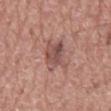No biopsy was performed on this lesion — it was imaged during a full skin examination and was not determined to be concerning. A male patient, approximately 75 years of age. A region of skin cropped from a whole-body photographic capture, roughly 15 mm wide. Located on the mid back. Longest diameter approximately 4.5 mm. The tile uses white-light illumination. Automated tile analysis of the lesion measured a border-irregularity index near 4/10 and a peripheral color-asymmetry measure near 2. And it measured an automated nevus-likeness rating near 0 out of 100 and a detector confidence of about 100 out of 100 that the crop contains a lesion.